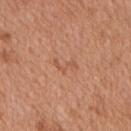biopsy status — catalogued during a skin exam; not biopsied
TBP lesion metrics — a footprint of about 2.5 mm², an outline eccentricity of about 0.9 (0 = round, 1 = elongated), and a symmetry-axis asymmetry near 0.6; a mean CIELAB color near L≈55 a*≈24 b*≈34, a lesion–skin lightness drop of about 6, and a normalized border contrast of about 4.5; a border-irregularity index near 8/10 and internal color variation of about 0 on a 0–10 scale; a classifier nevus-likeness of about 0/100 and lesion-presence confidence of about 100/100
imaging modality — ~15 mm tile from a whole-body skin photo
patient — male, in their mid- to late 60s
illumination — white-light
diameter — ~3 mm (longest diameter)
location — the mid back